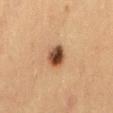Image and clinical context: The tile uses cross-polarized illumination. A lesion tile, about 15 mm wide, cut from a 3D total-body photograph. From the mid back. A female subject, aged 38 to 42. About 3 mm across.